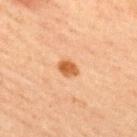Assessment:
The lesion was photographed on a routine skin check and not biopsied; there is no pathology result.
Clinical summary:
The lesion is on the back. A roughly 15 mm field-of-view crop from a total-body skin photograph. A male subject aged approximately 60. Captured under cross-polarized illumination. An algorithmic analysis of the crop reported an area of roughly 4 mm², a shape eccentricity near 0.65, and two-axis asymmetry of about 0.2. The analysis additionally found a border-irregularity rating of about 1.5/10, a within-lesion color-variation index near 2.5/10, and peripheral color asymmetry of about 1.